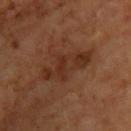Part of a total-body skin-imaging series; this lesion was reviewed on a skin check and was not flagged for biopsy. A male subject aged 63–67. Located on the upper back. A 15 mm crop from a total-body photograph taken for skin-cancer surveillance. About 7 mm across. The tile uses cross-polarized illumination. Automated image analysis of the tile measured about 7 CIELAB-L* units darker than the surrounding skin. It also reported internal color variation of about 3.5 on a 0–10 scale and a peripheral color-asymmetry measure near 1. The analysis additionally found a nevus-likeness score of about 5/100 and lesion-presence confidence of about 100/100.